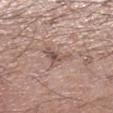Q: Is there a histopathology result?
A: no biopsy performed (imaged during a skin exam)
Q: How was this image acquired?
A: ~15 mm tile from a whole-body skin photo
Q: What lighting was used for the tile?
A: white-light
Q: How large is the lesion?
A: about 3.5 mm
Q: Who is the patient?
A: male, aged around 70
Q: What did automated image analysis measure?
A: an area of roughly 6 mm², a shape eccentricity near 0.5, and two-axis asymmetry of about 0.55; an automated nevus-likeness rating near 0 out of 100
Q: Where on the body is the lesion?
A: the left lower leg